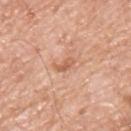Captured during whole-body skin photography for melanoma surveillance; the lesion was not biopsied. The subject is a male aged around 55. On the upper back. This image is a 15 mm lesion crop taken from a total-body photograph.